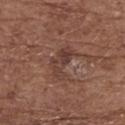Findings:
* workup · no biopsy performed (imaged during a skin exam)
* site · the back
* acquisition · 15 mm crop, total-body photography
* subject · female, roughly 75 years of age